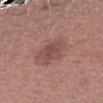notes: catalogued during a skin exam; not biopsied
image: total-body-photography crop, ~15 mm field of view
subject: female, aged approximately 45
anatomic site: the left forearm